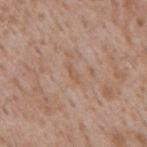<record>
  <biopsy_status>not biopsied; imaged during a skin examination</biopsy_status>
  <lesion_size>
    <long_diameter_mm_approx>3.0</long_diameter_mm_approx>
  </lesion_size>
  <lighting>white-light</lighting>
  <image>
    <source>total-body photography crop</source>
    <field_of_view_mm>15</field_of_view_mm>
  </image>
  <site>back</site>
  <patient>
    <sex>male</sex>
    <age_approx>45</age_approx>
  </patient>
</record>